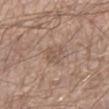notes = imaged on a skin check; not biopsied | image-analysis metrics = an average lesion color of about L≈54 a*≈16 b*≈26 (CIELAB), a lesion–skin lightness drop of about 7, and a normalized lesion–skin contrast near 5; border irregularity of about 2.5 on a 0–10 scale, a within-lesion color-variation index near 2.5/10, and radial color variation of about 1 | body site = the left forearm | acquisition = ~15 mm tile from a whole-body skin photo | subject = male, roughly 55 years of age | tile lighting = white-light illumination | size = ~3.5 mm (longest diameter).